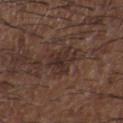Q: Is there a histopathology result?
A: total-body-photography surveillance lesion; no biopsy
Q: What lighting was used for the tile?
A: white-light illumination
Q: How was this image acquired?
A: ~15 mm crop, total-body skin-cancer survey
Q: What is the anatomic site?
A: the upper back
Q: Automated lesion metrics?
A: an area of roughly 6.5 mm², an outline eccentricity of about 0.6 (0 = round, 1 = elongated), and two-axis asymmetry of about 0.5; an average lesion color of about L≈29 a*≈16 b*≈19 (CIELAB), about 7 CIELAB-L* units darker than the surrounding skin, and a normalized border contrast of about 7.5; a border-irregularity index near 8.5/10, a within-lesion color-variation index near 3.5/10, and peripheral color asymmetry of about 1; a detector confidence of about 75 out of 100 that the crop contains a lesion
Q: Who is the patient?
A: male, roughly 50 years of age